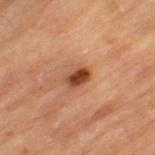<tbp_lesion>
  <biopsy_status>not biopsied; imaged during a skin examination</biopsy_status>
  <site>left thigh</site>
  <lighting>cross-polarized</lighting>
  <patient>
    <sex>male</sex>
    <age_approx>85+</age_approx>
  </patient>
  <image>
    <source>total-body photography crop</source>
    <field_of_view_mm>15</field_of_view_mm>
  </image>
  <lesion_size>
    <long_diameter_mm_approx>2.5</long_diameter_mm_approx>
  </lesion_size>
</tbp_lesion>